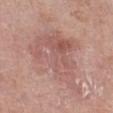Clinical impression: Recorded during total-body skin imaging; not selected for excision or biopsy. Acquisition and patient details: A 15 mm close-up tile from a total-body photography series done for melanoma screening. The total-body-photography lesion software estimated a lesion area of about 32 mm², a shape eccentricity near 0.65, and two-axis asymmetry of about 0.45. And it measured a mean CIELAB color near L≈57 a*≈22 b*≈23 and a lesion-to-skin contrast of about 5.5 (normalized; higher = more distinct). And it measured a border-irregularity index near 5.5/10. The analysis additionally found a classifier nevus-likeness of about 0/100 and a lesion-detection confidence of about 100/100. The subject is a female roughly 70 years of age. Measured at roughly 8.5 mm in maximum diameter. On the left lower leg.